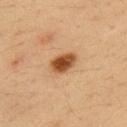biopsy_status: not biopsied; imaged during a skin examination
lesion_size:
  long_diameter_mm_approx: 3.5
site: left upper arm
lighting: cross-polarized
patient:
  sex: male
  age_approx: 35
automated_metrics:
  vs_skin_darker_L: 15.0
  color_variation_0_10: 4.0
  peripheral_color_asymmetry: 1.0
image:
  source: total-body photography crop
  field_of_view_mm: 15